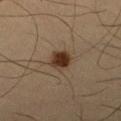– notes · imaged on a skin check; not biopsied
– anatomic site · the right lower leg
– automated metrics · a nevus-likeness score of about 100/100
– patient · male, aged around 35
– image · ~15 mm tile from a whole-body skin photo
– illumination · cross-polarized
– lesion diameter · about 3 mm